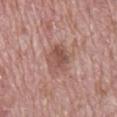Assessment: Recorded during total-body skin imaging; not selected for excision or biopsy. Background: This image is a 15 mm lesion crop taken from a total-body photograph. A male patient aged around 70. The lesion is located on the mid back.